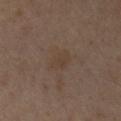Impression: Captured during whole-body skin photography for melanoma surveillance; the lesion was not biopsied. Acquisition and patient details: An algorithmic analysis of the crop reported a footprint of about 5 mm² and a shape eccentricity near 0.7. The software also gave border irregularity of about 2 on a 0–10 scale, a within-lesion color-variation index near 1.5/10, and radial color variation of about 0.5. It also reported an automated nevus-likeness rating near 0 out of 100 and lesion-presence confidence of about 100/100. The lesion is located on the left forearm. A male patient, about 55 years old. About 3 mm across. This is a cross-polarized tile. Cropped from a total-body skin-imaging series; the visible field is about 15 mm.